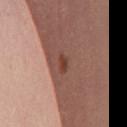Recorded during total-body skin imaging; not selected for excision or biopsy. About 2.5 mm across. An algorithmic analysis of the crop reported an average lesion color of about L≈42 a*≈23 b*≈26 (CIELAB) and a lesion–skin lightness drop of about 10. The analysis additionally found a border-irregularity rating of about 2.5/10, a within-lesion color-variation index near 0/10, and a peripheral color-asymmetry measure near 0. This is a white-light tile. A close-up tile cropped from a whole-body skin photograph, about 15 mm across. On the chest. A female patient, aged around 40.